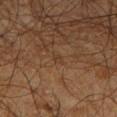Q: Is there a histopathology result?
A: total-body-photography surveillance lesion; no biopsy
Q: What did automated image analysis measure?
A: a border-irregularity rating of about 1.5/10, a color-variation rating of about 0/10, and peripheral color asymmetry of about 0
Q: What is the anatomic site?
A: the leg
Q: Patient demographics?
A: male, approximately 50 years of age
Q: What is the lesion's diameter?
A: about 1 mm
Q: Illumination type?
A: cross-polarized illumination
Q: What is the imaging modality?
A: ~15 mm tile from a whole-body skin photo